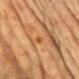Impression:
Recorded during total-body skin imaging; not selected for excision or biopsy.
Acquisition and patient details:
Measured at roughly 2.5 mm in maximum diameter. A female patient about 65 years old. Imaged with cross-polarized lighting. A 15 mm crop from a total-body photograph taken for skin-cancer surveillance. The lesion is on the chest.